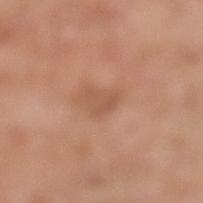Clinical impression:
This lesion was catalogued during total-body skin photography and was not selected for biopsy.
Background:
The subject is a male about 80 years old. This image is a 15 mm lesion crop taken from a total-body photograph. This is a white-light tile. Approximately 2.5 mm at its widest. Automated tile analysis of the lesion measured a within-lesion color-variation index near 1/10 and a peripheral color-asymmetry measure near 0.5. From the left lower leg.